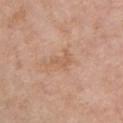  biopsy_status: not biopsied; imaged during a skin examination
  patient:
    sex: female
    age_approx: 75
  site: chest
  image:
    source: total-body photography crop
    field_of_view_mm: 15